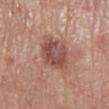Notes:
- notes — total-body-photography surveillance lesion; no biopsy
- subject — male, aged 68–72
- tile lighting — white-light illumination
- imaging modality — ~15 mm crop, total-body skin-cancer survey
- lesion diameter — ≈4 mm
- body site — the left lower leg